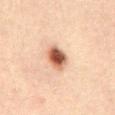Assessment:
This lesion was catalogued during total-body skin photography and was not selected for biopsy.
Context:
The lesion-visualizer software estimated a border-irregularity rating of about 2/10 and radial color variation of about 1.5. About 3.5 mm across. The subject is a male approximately 30 years of age. From the abdomen. Imaged with cross-polarized lighting. A 15 mm close-up tile from a total-body photography series done for melanoma screening.